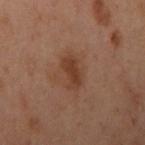• follow-up · imaged on a skin check; not biopsied
• illumination · cross-polarized
• image-analysis metrics · a lesion area of about 6 mm² and an outline eccentricity of about 0.85 (0 = round, 1 = elongated); a mean CIELAB color near L≈31 a*≈18 b*≈25 and about 7 CIELAB-L* units darker than the surrounding skin; an automated nevus-likeness rating near 40 out of 100 and a lesion-detection confidence of about 100/100
• lesion diameter · ~3.5 mm (longest diameter)
• site · the left arm
• image · total-body-photography crop, ~15 mm field of view
• subject · female, approximately 55 years of age